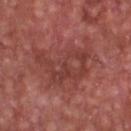No biopsy was performed on this lesion — it was imaged during a full skin examination and was not determined to be concerning.
The recorded lesion diameter is about 6.5 mm.
Located on the chest.
Captured under white-light illumination.
A 15 mm close-up tile from a total-body photography series done for melanoma screening.
A male subject approximately 65 years of age.